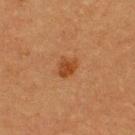workup: imaged on a skin check; not biopsied
patient: male, aged approximately 40
image: total-body-photography crop, ~15 mm field of view
location: the upper back